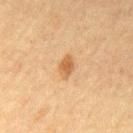Part of a total-body skin-imaging series; this lesion was reviewed on a skin check and was not flagged for biopsy. A male subject aged around 75. This is a cross-polarized tile. Measured at roughly 3 mm in maximum diameter. On the mid back. A 15 mm close-up tile from a total-body photography series done for melanoma screening. The total-body-photography lesion software estimated roughly 9 lightness units darker than nearby skin and a normalized border contrast of about 7.